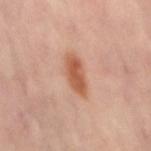Notes:
• workup · total-body-photography surveillance lesion; no biopsy
• imaging modality · 15 mm crop, total-body photography
• body site · the lower back
• subject · female, aged around 40
• lesion diameter · ≈5 mm
• lighting · cross-polarized